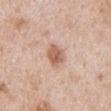Case summary:
* acquisition: total-body-photography crop, ~15 mm field of view
* diameter: about 3.5 mm
* subject: male, aged approximately 65
* automated lesion analysis: a shape eccentricity near 0.8; an average lesion color of about L≈61 a*≈21 b*≈30 (CIELAB), about 12 CIELAB-L* units darker than the surrounding skin, and a normalized border contrast of about 8; a nevus-likeness score of about 45/100 and lesion-presence confidence of about 100/100
* anatomic site: the chest
* lighting: white-light illumination